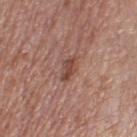workup: no biopsy performed (imaged during a skin exam)
subject: female, aged approximately 65
lighting: white-light
lesion size: about 3 mm
imaging modality: total-body-photography crop, ~15 mm field of view
site: the left leg